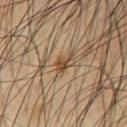biopsy status=no biopsy performed (imaged during a skin exam)
patient=male, about 40 years old
body site=the chest
image=total-body-photography crop, ~15 mm field of view
lighting=cross-polarized illumination
diameter=~3 mm (longest diameter)
image-analysis metrics=a lesion color around L≈48 a*≈16 b*≈33 in CIELAB, about 11 CIELAB-L* units darker than the surrounding skin, and a normalized border contrast of about 8; a border-irregularity rating of about 4/10 and radial color variation of about 0.5